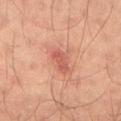No biopsy was performed on this lesion — it was imaged during a full skin examination and was not determined to be concerning. The recorded lesion diameter is about 3 mm. A male patient in their mid- to late 30s. The lesion-visualizer software estimated a mean CIELAB color near L≈52 a*≈28 b*≈28 and roughly 9 lightness units darker than nearby skin. The analysis additionally found radial color variation of about 0.5. It also reported a classifier nevus-likeness of about 0/100. The lesion is located on the back. A close-up tile cropped from a whole-body skin photograph, about 15 mm across. Imaged with cross-polarized lighting.